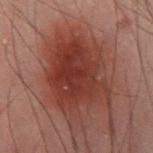Q: Is there a histopathology result?
A: catalogued during a skin exam; not biopsied
Q: What are the patient's age and sex?
A: male, aged 38–42
Q: What kind of image is this?
A: ~15 mm tile from a whole-body skin photo
Q: Where on the body is the lesion?
A: the right thigh
Q: Illumination type?
A: cross-polarized
Q: Lesion size?
A: about 9 mm